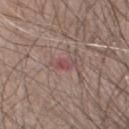| key | value |
|---|---|
| notes | imaged on a skin check; not biopsied |
| patient | male, aged around 80 |
| diameter | ≈3 mm |
| tile lighting | white-light |
| location | the head or neck |
| imaging modality | 15 mm crop, total-body photography |